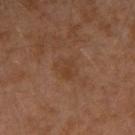No biopsy was performed on this lesion — it was imaged during a full skin examination and was not determined to be concerning. A male subject aged around 30. Cropped from a total-body skin-imaging series; the visible field is about 15 mm. The lesion-visualizer software estimated a lesion color around L≈38 a*≈19 b*≈29 in CIELAB, roughly 4 lightness units darker than nearby skin, and a lesion-to-skin contrast of about 4.5 (normalized; higher = more distinct). It also reported a classifier nevus-likeness of about 0/100 and a lesion-detection confidence of about 100/100. On the left arm. Measured at roughly 3 mm in maximum diameter.